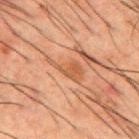follow-up — catalogued during a skin exam; not biopsied
tile lighting — cross-polarized illumination
subject — male, in their 50s
site — the upper back
image-analysis metrics — an area of roughly 6.5 mm², an eccentricity of roughly 0.9, and a symmetry-axis asymmetry near 0.5; a color-variation rating of about 3/10 and a peripheral color-asymmetry measure near 1
acquisition — 15 mm crop, total-body photography
lesion diameter — ~5 mm (longest diameter)